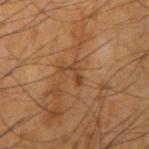workup — imaged on a skin check; not biopsied
tile lighting — cross-polarized illumination
TBP lesion metrics — an area of roughly 2.5 mm²
subject — male, in their mid- to late 60s
lesion size — ~3 mm (longest diameter)
anatomic site — the arm
imaging modality — ~15 mm crop, total-body skin-cancer survey